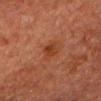Imaged during a routine full-body skin examination; the lesion was not biopsied and no histopathology is available.
A 15 mm close-up tile from a total-body photography series done for melanoma screening.
Approximately 3 mm at its widest.
This is a cross-polarized tile.
Located on the chest.
A male subject in their 80s.